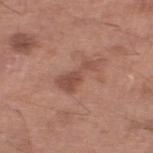notes=catalogued during a skin exam; not biopsied
lesion size=~5 mm (longest diameter)
anatomic site=the left lower leg
patient=male, aged 63 to 67
image source=~15 mm crop, total-body skin-cancer survey
automated metrics=a mean CIELAB color near L≈49 a*≈23 b*≈27, about 9 CIELAB-L* units darker than the surrounding skin, and a normalized lesion–skin contrast near 6.5; border irregularity of about 7 on a 0–10 scale, a color-variation rating of about 2/10, and radial color variation of about 0.5